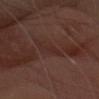workup: imaged on a skin check; not biopsied | automated lesion analysis: an area of roughly 4.5 mm² and two-axis asymmetry of about 0.35; about 5 CIELAB-L* units darker than the surrounding skin and a normalized lesion–skin contrast near 5; a nevus-likeness score of about 0/100 and a lesion-detection confidence of about 0/100 | location: the head or neck | tile lighting: white-light | lesion diameter: ≈4 mm | image source: 15 mm crop, total-body photography | patient: female, in their 50s.